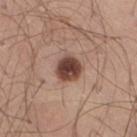Case summary:
– illumination — white-light illumination
– anatomic site — the right thigh
– subject — male, approximately 40 years of age
– imaging modality — ~15 mm tile from a whole-body skin photo
– lesion diameter — ≈3 mm